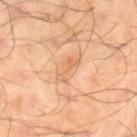notes=catalogued during a skin exam; not biopsied
subject=male, aged 63 to 67
image-analysis metrics=a mean CIELAB color near L≈62 a*≈22 b*≈36, a lesion–skin lightness drop of about 7, and a normalized border contrast of about 5
lighting=cross-polarized
lesion diameter=about 3.5 mm
image=15 mm crop, total-body photography
body site=the leg